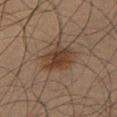{"biopsy_status": "not biopsied; imaged during a skin examination", "image": {"source": "total-body photography crop", "field_of_view_mm": 15}, "site": "right thigh", "lesion_size": {"long_diameter_mm_approx": 4.5}, "lighting": "cross-polarized", "patient": {"sex": "male", "age_approx": 65}}